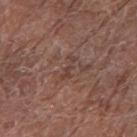No biopsy was performed on this lesion — it was imaged during a full skin examination and was not determined to be concerning.
A female patient approximately 80 years of age.
The total-body-photography lesion software estimated a lesion color around L≈41 a*≈19 b*≈23 in CIELAB, a lesion–skin lightness drop of about 7, and a lesion-to-skin contrast of about 5.5 (normalized; higher = more distinct). It also reported border irregularity of about 6.5 on a 0–10 scale and a within-lesion color-variation index near 0/10.
On the right forearm.
Cropped from a total-body skin-imaging series; the visible field is about 15 mm.
This is a white-light tile.